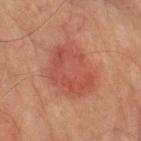Assessment: This lesion was catalogued during total-body skin photography and was not selected for biopsy. Clinical summary: The subject is a male aged 73–77. Automated tile analysis of the lesion measured an outline eccentricity of about 0.6 (0 = round, 1 = elongated) and a symmetry-axis asymmetry near 0.2. And it measured a lesion color around L≈43 a*≈24 b*≈26 in CIELAB and a normalized lesion–skin contrast near 5.5. It also reported an automated nevus-likeness rating near 70 out of 100. A 15 mm close-up extracted from a 3D total-body photography capture. Located on the left upper arm.